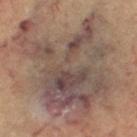The lesion was tiled from a total-body skin photograph and was not biopsied.
On the leg.
The tile uses cross-polarized illumination.
An algorithmic analysis of the crop reported an average lesion color of about L≈46 a*≈13 b*≈21 (CIELAB) and roughly 10 lightness units darker than nearby skin. And it measured border irregularity of about 7.5 on a 0–10 scale, a color-variation rating of about 8.5/10, and peripheral color asymmetry of about 2.5. It also reported a classifier nevus-likeness of about 0/100 and lesion-presence confidence of about 65/100.
A female patient, aged 63–67.
A 15 mm close-up extracted from a 3D total-body photography capture.
Longest diameter approximately 14 mm.